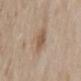{"biopsy_status": "not biopsied; imaged during a skin examination", "site": "back", "image": {"source": "total-body photography crop", "field_of_view_mm": 15}, "automated_metrics": {"area_mm2_approx": 5.5, "eccentricity": 0.6, "shape_asymmetry": 0.2, "vs_skin_darker_L": 9.0, "vs_skin_contrast_norm": 6.5, "border_irregularity_0_10": 2.0, "color_variation_0_10": 2.0, "peripheral_color_asymmetry": 0.5}, "lesion_size": {"long_diameter_mm_approx": 3.0}, "patient": {"sex": "female", "age_approx": 60}, "lighting": "white-light"}